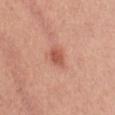Notes:
– workup · catalogued during a skin exam; not biopsied
– location · the abdomen
– subject · female, roughly 25 years of age
– illumination · white-light illumination
– lesion size · about 2.5 mm
– automated lesion analysis · an area of roughly 3.5 mm², an eccentricity of roughly 0.7, and a symmetry-axis asymmetry near 0.15; an automated nevus-likeness rating near 95 out of 100
– image source · ~15 mm tile from a whole-body skin photo